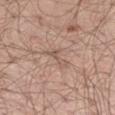biopsy_status: not biopsied; imaged during a skin examination
site: leg
patient:
  sex: male
  age_approx: 65
image:
  source: total-body photography crop
  field_of_view_mm: 15